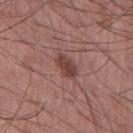Imaged during a routine full-body skin examination; the lesion was not biopsied and no histopathology is available. A male patient aged around 60. This image is a 15 mm lesion crop taken from a total-body photograph. Automated image analysis of the tile measured a lesion area of about 5 mm² and an eccentricity of roughly 0.85. The software also gave a mean CIELAB color near L≈42 a*≈23 b*≈22, a lesion–skin lightness drop of about 11, and a lesion-to-skin contrast of about 8.5 (normalized; higher = more distinct). This is a white-light tile. The lesion is on the front of the torso.